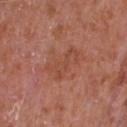biopsy status=imaged on a skin check; not biopsied | anatomic site=the chest | acquisition=~15 mm tile from a whole-body skin photo | lesion size=≈4.5 mm | automated lesion analysis=an area of roughly 6.5 mm², a shape eccentricity near 0.9, and a symmetry-axis asymmetry near 0.5; about 6 CIELAB-L* units darker than the surrounding skin | lighting=white-light illumination | subject=male, about 65 years old.